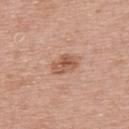notes — no biopsy performed (imaged during a skin exam)
acquisition — ~15 mm tile from a whole-body skin photo
lighting — white-light illumination
subject — male, approximately 65 years of age
anatomic site — the upper back
size — about 3 mm